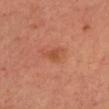{
  "lighting": "cross-polarized",
  "site": "chest",
  "image": {
    "source": "total-body photography crop",
    "field_of_view_mm": 15
  },
  "automated_metrics": {
    "cielab_L": 48,
    "cielab_a": 27,
    "cielab_b": 35,
    "vs_skin_contrast_norm": 6.0,
    "nevus_likeness_0_100": 5
  },
  "lesion_size": {
    "long_diameter_mm_approx": 2.5
  },
  "patient": {
    "sex": "female",
    "age_approx": 45
  }
}